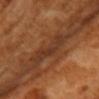Captured during whole-body skin photography for melanoma surveillance; the lesion was not biopsied. The lesion is on the left upper arm. The lesion-visualizer software estimated an area of roughly 10 mm², an eccentricity of roughly 0.85, and a symmetry-axis asymmetry near 0.35. It also reported a nevus-likeness score of about 0/100 and lesion-presence confidence of about 55/100. A 15 mm close-up tile from a total-body photography series done for melanoma screening. The lesion's longest dimension is about 4.5 mm. The tile uses cross-polarized illumination. A female subject, about 55 years old.